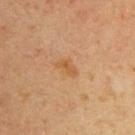Notes:
- follow-up · total-body-photography surveillance lesion; no biopsy
- body site · the upper back
- automated metrics · a footprint of about 3.5 mm² and an eccentricity of roughly 0.85; internal color variation of about 2 on a 0–10 scale and a peripheral color-asymmetry measure near 0.5; an automated nevus-likeness rating near 0 out of 100 and a lesion-detection confidence of about 100/100
- image source · ~15 mm crop, total-body skin-cancer survey
- diameter · about 3 mm
- patient · female, approximately 40 years of age
- lighting · cross-polarized